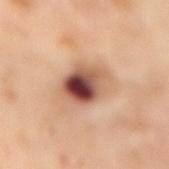Impression:
This lesion was catalogued during total-body skin photography and was not selected for biopsy.
Context:
Automated image analysis of the tile measured a lesion color around L≈52 a*≈24 b*≈29 in CIELAB and about 20 CIELAB-L* units darker than the surrounding skin. Measured at roughly 5 mm in maximum diameter. A 15 mm close-up extracted from a 3D total-body photography capture. The lesion is on the mid back. The patient is a female aged 53–57.